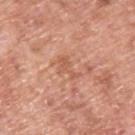Imaged during a routine full-body skin examination; the lesion was not biopsied and no histopathology is available. A 15 mm crop from a total-body photograph taken for skin-cancer surveillance. The subject is a male aged approximately 55. The tile uses white-light illumination. Located on the upper back. Approximately 3 mm at its widest.